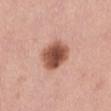Q: What is the anatomic site?
A: the leg
Q: What is the imaging modality?
A: 15 mm crop, total-body photography
Q: What is the lesion's diameter?
A: ≈4 mm
Q: Patient demographics?
A: female, in their mid-40s